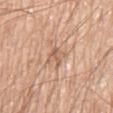Assessment: Part of a total-body skin-imaging series; this lesion was reviewed on a skin check and was not flagged for biopsy. Clinical summary: The total-body-photography lesion software estimated an area of roughly 4.5 mm² and an eccentricity of roughly 0.55. The analysis additionally found a mean CIELAB color near L≈61 a*≈20 b*≈32, a lesion–skin lightness drop of about 9, and a normalized border contrast of about 6. It also reported a classifier nevus-likeness of about 0/100 and a lesion-detection confidence of about 75/100. On the chest. Longest diameter approximately 2.5 mm. A male patient, approximately 80 years of age. Cropped from a total-body skin-imaging series; the visible field is about 15 mm. The tile uses white-light illumination.